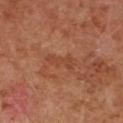The lesion was tiled from a total-body skin photograph and was not biopsied. Located on the right upper arm. About 3.5 mm across. This image is a 15 mm lesion crop taken from a total-body photograph. Automated image analysis of the tile measured a border-irregularity rating of about 6.5/10 and a color-variation rating of about 0/10. The tile uses cross-polarized illumination. A female patient aged 48–52.